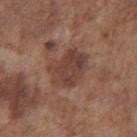Assessment:
No biopsy was performed on this lesion — it was imaged during a full skin examination and was not determined to be concerning.
Image and clinical context:
A roughly 15 mm field-of-view crop from a total-body skin photograph. The lesion is on the chest. A male patient aged 73–77. Approximately 5 mm at its widest. An algorithmic analysis of the crop reported an area of roughly 14 mm². The software also gave a mean CIELAB color near L≈41 a*≈19 b*≈25 and about 9 CIELAB-L* units darker than the surrounding skin. This is a white-light tile.